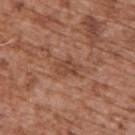Q: Is there a histopathology result?
A: imaged on a skin check; not biopsied
Q: What kind of image is this?
A: ~15 mm crop, total-body skin-cancer survey
Q: How large is the lesion?
A: about 3 mm
Q: What are the patient's age and sex?
A: male, approximately 75 years of age
Q: Where on the body is the lesion?
A: the upper back